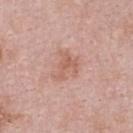The lesion was tiled from a total-body skin photograph and was not biopsied. Measured at roughly 3.5 mm in maximum diameter. From the back. Imaged with white-light lighting. The total-body-photography lesion software estimated a shape eccentricity near 0.65 and a symmetry-axis asymmetry near 0.5. The software also gave a nevus-likeness score of about 0/100 and a detector confidence of about 100 out of 100 that the crop contains a lesion. A female patient in their 30s. A 15 mm close-up tile from a total-body photography series done for melanoma screening.